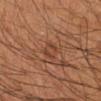The tile uses cross-polarized illumination. On the left arm. Automated image analysis of the tile measured an area of roughly 5 mm², an eccentricity of roughly 0.75, and two-axis asymmetry of about 0.25. And it measured a mean CIELAB color near L≈42 a*≈22 b*≈31, roughly 7 lightness units darker than nearby skin, and a normalized lesion–skin contrast near 5.5. The software also gave border irregularity of about 2 on a 0–10 scale and a peripheral color-asymmetry measure near 0.5. The lesion's longest dimension is about 3 mm. A male patient roughly 50 years of age. This image is a 15 mm lesion crop taken from a total-body photograph.